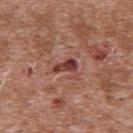Captured during whole-body skin photography for melanoma surveillance; the lesion was not biopsied. Longest diameter approximately 3 mm. A male subject, approximately 45 years of age. A 15 mm crop from a total-body photograph taken for skin-cancer surveillance. This is a white-light tile. Automated image analysis of the tile measured a lesion area of about 3.5 mm², a shape eccentricity near 0.9, and a symmetry-axis asymmetry near 0.35. It also reported an average lesion color of about L≈39 a*≈28 b*≈24 (CIELAB) and a lesion–skin lightness drop of about 14. The analysis additionally found a border-irregularity rating of about 3.5/10 and radial color variation of about 0.5. It also reported a nevus-likeness score of about 0/100 and a detector confidence of about 100 out of 100 that the crop contains a lesion. From the upper back.